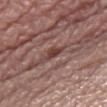Imaged during a routine full-body skin examination; the lesion was not biopsied and no histopathology is available. Located on the right thigh. The lesion's longest dimension is about 2.5 mm. The tile uses white-light illumination. A 15 mm close-up tile from a total-body photography series done for melanoma screening. The subject is a female in their mid-60s.